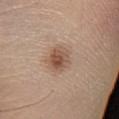– follow-up — imaged on a skin check; not biopsied
– location — the left lower leg
– subject — male, in their mid- to late 60s
– illumination — white-light
– size — ~3.5 mm (longest diameter)
– acquisition — total-body-photography crop, ~15 mm field of view